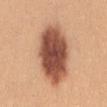illumination: white-light
site: the back
patient: female, approximately 25 years of age
image-analysis metrics: a footprint of about 32 mm², an outline eccentricity of about 0.85 (0 = round, 1 = elongated), and a symmetry-axis asymmetry near 0.1; a mean CIELAB color near L≈53 a*≈24 b*≈31, roughly 20 lightness units darker than nearby skin, and a normalized border contrast of about 12.5; an automated nevus-likeness rating near 100 out of 100 and a lesion-detection confidence of about 100/100
imaging modality: total-body-photography crop, ~15 mm field of view